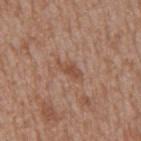Notes:
• workup — catalogued during a skin exam; not biopsied
• illumination — white-light illumination
• automated metrics — a lesion area of about 3.5 mm², a shape eccentricity near 0.9, and two-axis asymmetry of about 0.25; a border-irregularity index near 3/10 and a peripheral color-asymmetry measure near 0
• patient — male, aged 63–67
• body site — the mid back
• acquisition — total-body-photography crop, ~15 mm field of view